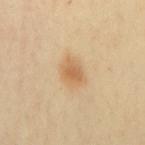notes — total-body-photography surveillance lesion; no biopsy
subject — female, aged around 40
site — the mid back
acquisition — 15 mm crop, total-body photography